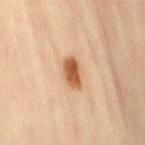{
  "image": {
    "source": "total-body photography crop",
    "field_of_view_mm": 15
  },
  "site": "leg",
  "lesion_size": {
    "long_diameter_mm_approx": 3.5
  },
  "patient": {
    "sex": "female",
    "age_approx": 45
  }
}